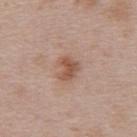Assessment:
Captured during whole-body skin photography for melanoma surveillance; the lesion was not biopsied.
Clinical summary:
The tile uses white-light illumination. About 3 mm across. From the upper back. Automated image analysis of the tile measured an average lesion color of about L≈54 a*≈20 b*≈28 (CIELAB), roughly 10 lightness units darker than nearby skin, and a normalized border contrast of about 7. And it measured a border-irregularity index near 4/10, a color-variation rating of about 2.5/10, and peripheral color asymmetry of about 1. A roughly 15 mm field-of-view crop from a total-body skin photograph. A female patient, roughly 50 years of age.